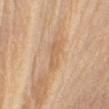The lesion was tiled from a total-body skin photograph and was not biopsied. A close-up tile cropped from a whole-body skin photograph, about 15 mm across. The lesion-visualizer software estimated an average lesion color of about L≈63 a*≈19 b*≈36 (CIELAB). The analysis additionally found a classifier nevus-likeness of about 0/100. The lesion is on the right upper arm. Imaged with white-light lighting. The patient is a male aged 68 to 72. The lesion's longest dimension is about 3.5 mm.